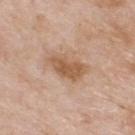Part of a total-body skin-imaging series; this lesion was reviewed on a skin check and was not flagged for biopsy.
A region of skin cropped from a whole-body photographic capture, roughly 15 mm wide.
Located on the upper back.
The tile uses white-light illumination.
A male patient approximately 60 years of age.